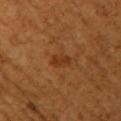Q: Was a biopsy performed?
A: imaged on a skin check; not biopsied
Q: Who is the patient?
A: female, aged 53–57
Q: What is the imaging modality?
A: ~15 mm crop, total-body skin-cancer survey
Q: What did automated image analysis measure?
A: a detector confidence of about 100 out of 100 that the crop contains a lesion
Q: Where on the body is the lesion?
A: the right upper arm